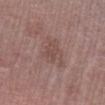automated lesion analysis = a lesion area of about 10 mm² and an eccentricity of roughly 0.75; an average lesion color of about L≈49 a*≈18 b*≈22 (CIELAB), a lesion–skin lightness drop of about 6, and a normalized lesion–skin contrast near 5; a border-irregularity index near 3.5/10, a color-variation rating of about 2.5/10, and peripheral color asymmetry of about 1; a nevus-likeness score of about 0/100 and a lesion-detection confidence of about 100/100 | patient = female, aged 63–67 | tile lighting = white-light illumination | body site = the leg | image source = total-body-photography crop, ~15 mm field of view | diameter = ≈4.5 mm.